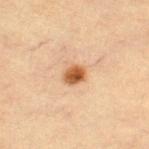Part of a total-body skin-imaging series; this lesion was reviewed on a skin check and was not flagged for biopsy. A lesion tile, about 15 mm wide, cut from a 3D total-body photograph. On the right thigh. The tile uses cross-polarized illumination. A female subject about 65 years old.